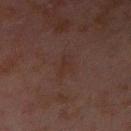The lesion was photographed on a routine skin check and not biopsied; there is no pathology result. Automated tile analysis of the lesion measured an area of roughly 3.5 mm², an outline eccentricity of about 0.9 (0 = round, 1 = elongated), and a symmetry-axis asymmetry near 0.4. The analysis additionally found an average lesion color of about L≈23 a*≈14 b*≈18 (CIELAB) and about 3 CIELAB-L* units darker than the surrounding skin. It also reported border irregularity of about 3.5 on a 0–10 scale, internal color variation of about 1 on a 0–10 scale, and radial color variation of about 0. Located on the left forearm. Imaged with cross-polarized lighting. A male patient, aged 28 to 32. About 3 mm across. Cropped from a whole-body photographic skin survey; the tile spans about 15 mm.